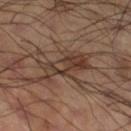Image and clinical context:
Cropped from a whole-body photographic skin survey; the tile spans about 15 mm. A male patient aged around 60. The lesion is on the right thigh. Measured at roughly 6 mm in maximum diameter. Automated tile analysis of the lesion measured an automated nevus-likeness rating near 0 out of 100 and a detector confidence of about 95 out of 100 that the crop contains a lesion. Imaged with cross-polarized lighting.